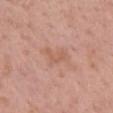| feature | finding |
|---|---|
| biopsy status | total-body-photography surveillance lesion; no biopsy |
| lesion diameter | ≈3.5 mm |
| image | ~15 mm tile from a whole-body skin photo |
| tile lighting | white-light |
| anatomic site | the mid back |
| patient | female, roughly 65 years of age |
| TBP lesion metrics | a shape eccentricity near 0.85 and a shape-asymmetry score of about 0.45 (0 = symmetric); a lesion–skin lightness drop of about 6 and a normalized border contrast of about 5; an automated nevus-likeness rating near 0 out of 100 |